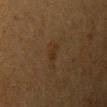Assessment:
Captured during whole-body skin photography for melanoma surveillance; the lesion was not biopsied.
Image and clinical context:
Cropped from a whole-body photographic skin survey; the tile spans about 15 mm. The subject is a female aged approximately 40. On the left upper arm.